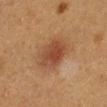<tbp_lesion>
  <biopsy_status>not biopsied; imaged during a skin examination</biopsy_status>
  <lighting>cross-polarized</lighting>
  <site>left lower leg</site>
  <lesion_size>
    <long_diameter_mm_approx>4.5</long_diameter_mm_approx>
  </lesion_size>
  <automated_metrics>
    <area_mm2_approx>12.0</area_mm2_approx>
    <eccentricity>0.7</eccentricity>
    <border_irregularity_0_10>2.0</border_irregularity_0_10>
    <color_variation_0_10>3.5</color_variation_0_10>
    <peripheral_color_asymmetry>1.0</peripheral_color_asymmetry>
    <nevus_likeness_0_100>95</nevus_likeness_0_100>
    <lesion_detection_confidence_0_100>100</lesion_detection_confidence_0_100>
  </automated_metrics>
  <patient>
    <sex>female</sex>
    <age_approx>40</age_approx>
  </patient>
  <image>
    <source>total-body photography crop</source>
    <field_of_view_mm>15</field_of_view_mm>
  </image>
</tbp_lesion>